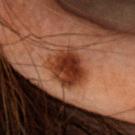  biopsy_status: not biopsied; imaged during a skin examination
  image:
    source: total-body photography crop
    field_of_view_mm: 15
  site: head or neck
  patient:
    sex: female
    age_approx: 55